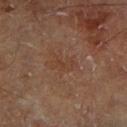The lesion was tiled from a total-body skin photograph and was not biopsied.
A 15 mm close-up tile from a total-body photography series done for melanoma screening.
The subject is a male in their 70s.
Imaged with cross-polarized lighting.
From the right lower leg.